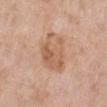Q: Was a biopsy performed?
A: catalogued during a skin exam; not biopsied
Q: Who is the patient?
A: female, in their 70s
Q: What is the anatomic site?
A: the left lower leg
Q: Automated lesion metrics?
A: an outline eccentricity of about 0.65 (0 = round, 1 = elongated); a within-lesion color-variation index near 5/10 and radial color variation of about 2; a nevus-likeness score of about 0/100
Q: What kind of image is this?
A: 15 mm crop, total-body photography
Q: Lesion size?
A: ≈5 mm